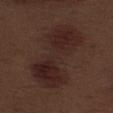Captured during whole-body skin photography for melanoma surveillance; the lesion was not biopsied.
A male subject, aged 68–72.
Located on the left thigh.
A 15 mm close-up extracted from a 3D total-body photography capture.